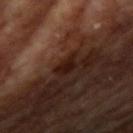This lesion was catalogued during total-body skin photography and was not selected for biopsy. Captured under cross-polarized illumination. A male patient aged around 65. A close-up tile cropped from a whole-body skin photograph, about 15 mm across. The lesion-visualizer software estimated a footprint of about 4 mm², an outline eccentricity of about 0.8 (0 = round, 1 = elongated), and two-axis asymmetry of about 0.3. And it measured internal color variation of about 1 on a 0–10 scale. The software also gave a classifier nevus-likeness of about 45/100. From the right upper arm.